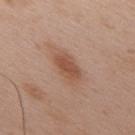Part of a total-body skin-imaging series; this lesion was reviewed on a skin check and was not flagged for biopsy. The lesion is on the upper back. A male patient approximately 50 years of age. A roughly 15 mm field-of-view crop from a total-body skin photograph.